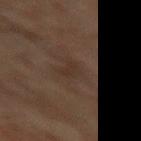No biopsy was performed on this lesion — it was imaged during a full skin examination and was not determined to be concerning.
Automated tile analysis of the lesion measured a mean CIELAB color near L≈22 a*≈11 b*≈17, a lesion–skin lightness drop of about 4, and a lesion-to-skin contrast of about 5 (normalized; higher = more distinct).
The lesion's longest dimension is about 2.5 mm.
A male patient, aged 68–72.
A 15 mm close-up extracted from a 3D total-body photography capture.
Located on the right thigh.